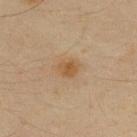biopsy_status: not biopsied; imaged during a skin examination
lesion_size:
  long_diameter_mm_approx: 2.5
automated_metrics:
  area_mm2_approx: 4.0
  cielab_L: 47
  cielab_a: 15
  cielab_b: 34
  vs_skin_darker_L: 7.0
  vs_skin_contrast_norm: 7.5
  border_irregularity_0_10: 2.5
  peripheral_color_asymmetry: 0.5
  nevus_likeness_0_100: 65
  lesion_detection_confidence_0_100: 100
image:
  source: total-body photography crop
  field_of_view_mm: 15
lighting: cross-polarized
site: upper back
patient:
  sex: male
  age_approx: 35